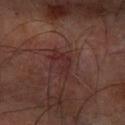notes: total-body-photography surveillance lesion; no biopsy | imaging modality: 15 mm crop, total-body photography | subject: male, in their mid- to late 60s | location: the left forearm | illumination: cross-polarized illumination | automated metrics: an area of roughly 5 mm², an eccentricity of roughly 0.85, and a symmetry-axis asymmetry near 0.45; a classifier nevus-likeness of about 5/100.